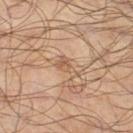Assessment:
Captured during whole-body skin photography for melanoma surveillance; the lesion was not biopsied.